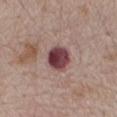Acquisition and patient details:
Located on the mid back. The recorded lesion diameter is about 3.5 mm. The total-body-photography lesion software estimated border irregularity of about 1 on a 0–10 scale and a peripheral color-asymmetry measure near 2.5. It also reported a nevus-likeness score of about 15/100 and lesion-presence confidence of about 100/100. The tile uses white-light illumination. The patient is a male in their mid- to late 60s. Cropped from a total-body skin-imaging series; the visible field is about 15 mm.